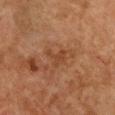Case summary:
• notes — imaged on a skin check; not biopsied
• imaging modality — ~15 mm tile from a whole-body skin photo
• site — the chest
• lighting — cross-polarized illumination
• patient — female, aged around 60
• lesion size — about 2.5 mm